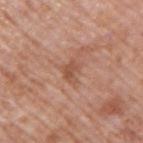No biopsy was performed on this lesion — it was imaged during a full skin examination and was not determined to be concerning.